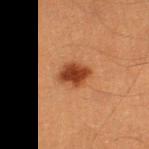Clinical impression: The lesion was tiled from a total-body skin photograph and was not biopsied. Background: A close-up tile cropped from a whole-body skin photograph, about 15 mm across. From the left thigh. The tile uses cross-polarized illumination. Automated tile analysis of the lesion measured an outline eccentricity of about 0.65 (0 = round, 1 = elongated) and two-axis asymmetry of about 0.2. The software also gave an average lesion color of about L≈35 a*≈24 b*≈32 (CIELAB) and a lesion-to-skin contrast of about 11 (normalized; higher = more distinct). The analysis additionally found border irregularity of about 2 on a 0–10 scale, internal color variation of about 3 on a 0–10 scale, and peripheral color asymmetry of about 1. About 3 mm across. The patient is a male roughly 40 years of age.